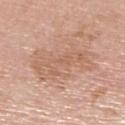Part of a total-body skin-imaging series; this lesion was reviewed on a skin check and was not flagged for biopsy. A lesion tile, about 15 mm wide, cut from a 3D total-body photograph. A female patient in their 70s. From the right lower leg.